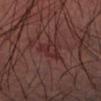Acquisition and patient details:
A male subject, aged around 60. Cropped from a total-body skin-imaging series; the visible field is about 15 mm. An algorithmic analysis of the crop reported a footprint of about 6 mm², an outline eccentricity of about 0.5 (0 = round, 1 = elongated), and a shape-asymmetry score of about 0.4 (0 = symmetric). The analysis additionally found an average lesion color of about L≈25 a*≈21 b*≈17 (CIELAB), about 5 CIELAB-L* units darker than the surrounding skin, and a lesion-to-skin contrast of about 5.5 (normalized; higher = more distinct). The software also gave border irregularity of about 3.5 on a 0–10 scale, a color-variation rating of about 4/10, and radial color variation of about 1.5. It also reported lesion-presence confidence of about 90/100. Longest diameter approximately 3 mm. The lesion is located on the left forearm.